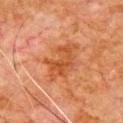notes — total-body-photography surveillance lesion; no biopsy
patient — male, aged 78 to 82
location — the chest
lighting — cross-polarized illumination
acquisition — 15 mm crop, total-body photography